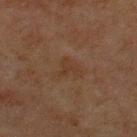Part of a total-body skin-imaging series; this lesion was reviewed on a skin check and was not flagged for biopsy. A male subject, in their mid- to late 60s. The total-body-photography lesion software estimated border irregularity of about 6.5 on a 0–10 scale, internal color variation of about 0 on a 0–10 scale, and radial color variation of about 0. A lesion tile, about 15 mm wide, cut from a 3D total-body photograph. The lesion is located on the front of the torso. Captured under cross-polarized illumination.